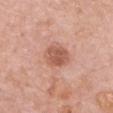Clinical impression: The lesion was tiled from a total-body skin photograph and was not biopsied. Background: A 15 mm crop from a total-body photograph taken for skin-cancer surveillance. An algorithmic analysis of the crop reported a border-irregularity index near 1.5/10, a color-variation rating of about 3/10, and peripheral color asymmetry of about 1. Longest diameter approximately 3.5 mm. This is a white-light tile. From the chest. A female subject aged approximately 60.